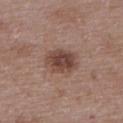biopsy status = imaged on a skin check; not biopsied
tile lighting = white-light illumination
location = the upper back
imaging modality = 15 mm crop, total-body photography
size = ~4 mm (longest diameter)
patient = female, aged 63 to 67